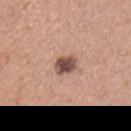Imaged during a routine full-body skin examination; the lesion was not biopsied and no histopathology is available. The lesion is on the chest. Captured under white-light illumination. Approximately 3 mm at its widest. A 15 mm close-up tile from a total-body photography series done for melanoma screening. A male patient in their mid- to late 40s.